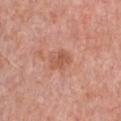Impression: Part of a total-body skin-imaging series; this lesion was reviewed on a skin check and was not flagged for biopsy. Background: The lesion is located on the chest. The lesion's longest dimension is about 2.5 mm. The patient is a female aged around 65. This image is a 15 mm lesion crop taken from a total-body photograph. The tile uses white-light illumination.